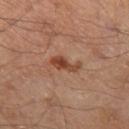Recorded during total-body skin imaging; not selected for excision or biopsy. Imaged with cross-polarized lighting. The subject is a male aged 63–67. The lesion-visualizer software estimated an area of roughly 4.5 mm², a shape eccentricity near 0.9, and a symmetry-axis asymmetry near 0.4. And it measured lesion-presence confidence of about 100/100. The lesion is located on the left thigh. This image is a 15 mm lesion crop taken from a total-body photograph.